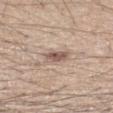Findings:
* image source · ~15 mm crop, total-body skin-cancer survey
* anatomic site · the right forearm
* diameter · about 2.5 mm
* automated metrics · a footprint of about 5.5 mm² and an eccentricity of roughly 0.55; a border-irregularity index near 2.5/10; a lesion-detection confidence of about 100/100
* subject · male, about 30 years old